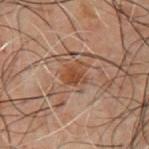Impression:
Part of a total-body skin-imaging series; this lesion was reviewed on a skin check and was not flagged for biopsy.
Clinical summary:
A male patient aged 48 to 52. On the front of the torso. Measured at roughly 3 mm in maximum diameter. Imaged with cross-polarized lighting. An algorithmic analysis of the crop reported an area of roughly 5 mm², an outline eccentricity of about 0.55 (0 = round, 1 = elongated), and a symmetry-axis asymmetry near 0.3. The software also gave a nevus-likeness score of about 15/100 and a detector confidence of about 100 out of 100 that the crop contains a lesion. A lesion tile, about 15 mm wide, cut from a 3D total-body photograph.